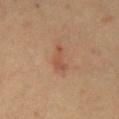{
  "biopsy_status": "not biopsied; imaged during a skin examination",
  "lighting": "cross-polarized",
  "lesion_size": {
    "long_diameter_mm_approx": 4.0
  },
  "site": "chest",
  "patient": {
    "sex": "male",
    "age_approx": 70
  },
  "image": {
    "source": "total-body photography crop",
    "field_of_view_mm": 15
  }
}